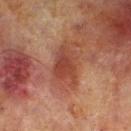Assessment:
Recorded during total-body skin imaging; not selected for excision or biopsy.